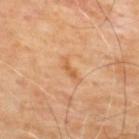Captured during whole-body skin photography for melanoma surveillance; the lesion was not biopsied. About 2.5 mm across. From the back. Captured under cross-polarized illumination. The total-body-photography lesion software estimated an area of roughly 2 mm² and a symmetry-axis asymmetry near 0.45. The software also gave a border-irregularity index near 4.5/10 and a within-lesion color-variation index near 0/10. The subject is a male roughly 65 years of age. A 15 mm crop from a total-body photograph taken for skin-cancer surveillance.